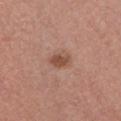This lesion was catalogued during total-body skin photography and was not selected for biopsy.
The subject is a female aged 23 to 27.
A 15 mm close-up tile from a total-body photography series done for melanoma screening.
From the front of the torso.